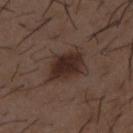notes: total-body-photography surveillance lesion; no biopsy
lesion size: about 5 mm
image source: 15 mm crop, total-body photography
patient: male, roughly 50 years of age
TBP lesion metrics: a shape eccentricity near 0.8 and a symmetry-axis asymmetry near 0.2; a lesion color around L≈27 a*≈16 b*≈20 in CIELAB, about 11 CIELAB-L* units darker than the surrounding skin, and a lesion-to-skin contrast of about 11 (normalized; higher = more distinct); a classifier nevus-likeness of about 90/100 and lesion-presence confidence of about 100/100
tile lighting: white-light
location: the back